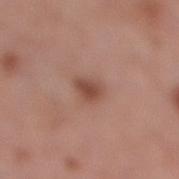{"biopsy_status": "not biopsied; imaged during a skin examination", "patient": {"sex": "female", "age_approx": 55}, "site": "right lower leg", "image": {"source": "total-body photography crop", "field_of_view_mm": 15}}